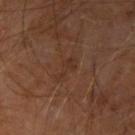workup: no biopsy performed (imaged during a skin exam)
automated lesion analysis: a lesion color around L≈29 a*≈18 b*≈25 in CIELAB and a lesion-to-skin contrast of about 5 (normalized; higher = more distinct); a classifier nevus-likeness of about 0/100 and a detector confidence of about 100 out of 100 that the crop contains a lesion
anatomic site: the left forearm
size: ≈2.5 mm
patient: male, about 65 years old
image source: ~15 mm tile from a whole-body skin photo
tile lighting: cross-polarized illumination